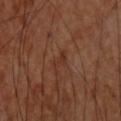Q: Is there a histopathology result?
A: no biopsy performed (imaged during a skin exam)
Q: What is the lesion's diameter?
A: ~2.5 mm (longest diameter)
Q: What is the anatomic site?
A: the upper back
Q: Who is the patient?
A: male, approximately 60 years of age
Q: How was this image acquired?
A: total-body-photography crop, ~15 mm field of view
Q: What did automated image analysis measure?
A: a mean CIELAB color near L≈32 a*≈21 b*≈27, roughly 5 lightness units darker than nearby skin, and a normalized lesion–skin contrast near 5.5; an automated nevus-likeness rating near 0 out of 100